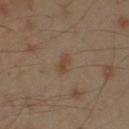Captured during whole-body skin photography for melanoma surveillance; the lesion was not biopsied. Cropped from a whole-body photographic skin survey; the tile spans about 15 mm. The lesion is located on the arm. A male patient aged 43 to 47. About 2.5 mm across. Automated image analysis of the tile measured a footprint of about 2.5 mm², an eccentricity of roughly 0.85, and a shape-asymmetry score of about 0.25 (0 = symmetric). The analysis additionally found an average lesion color of about L≈42 a*≈15 b*≈28 (CIELAB) and a lesion-to-skin contrast of about 6 (normalized; higher = more distinct). This is a cross-polarized tile.